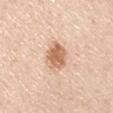This lesion was catalogued during total-body skin photography and was not selected for biopsy. An algorithmic analysis of the crop reported a footprint of about 8 mm², an eccentricity of roughly 0.3, and a symmetry-axis asymmetry near 0.15. The software also gave a mean CIELAB color near L≈65 a*≈21 b*≈34, roughly 14 lightness units darker than nearby skin, and a lesion-to-skin contrast of about 8.5 (normalized; higher = more distinct). Located on the left upper arm. A roughly 15 mm field-of-view crop from a total-body skin photograph. The patient is a male aged approximately 45. The recorded lesion diameter is about 3.5 mm. This is a white-light tile.